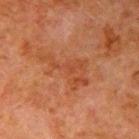Q: Was a biopsy performed?
A: no biopsy performed (imaged during a skin exam)
Q: How was this image acquired?
A: ~15 mm crop, total-body skin-cancer survey
Q: How large is the lesion?
A: ≈6 mm
Q: Lesion location?
A: the right upper arm
Q: Patient demographics?
A: male, about 80 years old